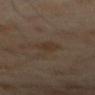Notes:
• biopsy status: imaged on a skin check; not biopsied
• imaging modality: 15 mm crop, total-body photography
• patient: male, in their mid-60s
• illumination: cross-polarized illumination